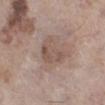Context:
This is a white-light tile. The lesion's longest dimension is about 4 mm. A female subject approximately 75 years of age. A 15 mm crop from a total-body photograph taken for skin-cancer surveillance. Automated tile analysis of the lesion measured a border-irregularity index near 4/10, a color-variation rating of about 3.5/10, and peripheral color asymmetry of about 1. On the right lower leg.